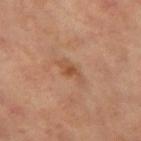Captured during whole-body skin photography for melanoma surveillance; the lesion was not biopsied. The total-body-photography lesion software estimated a lesion area of about 4 mm². The software also gave a lesion color around L≈50 a*≈21 b*≈33 in CIELAB and a normalized lesion–skin contrast near 6.5. A 15 mm crop from a total-body photograph taken for skin-cancer surveillance. Imaged with cross-polarized lighting. A female subject, in their mid-60s. From the left thigh.